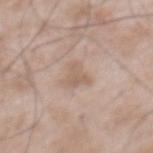<tbp_lesion>
<biopsy_status>not biopsied; imaged during a skin examination</biopsy_status>
<patient>
  <sex>male</sex>
  <age_approx>50</age_approx>
</patient>
<lighting>white-light</lighting>
<site>mid back</site>
<automated_metrics>
  <area_mm2_approx>4.0</area_mm2_approx>
  <eccentricity>0.7</eccentricity>
  <shape_asymmetry>0.5</shape_asymmetry>
  <cielab_L>59</cielab_L>
  <cielab_a>16</cielab_a>
  <cielab_b>27</cielab_b>
  <vs_skin_contrast_norm>5.5</vs_skin_contrast_norm>
  <border_irregularity_0_10>5.0</border_irregularity_0_10>
  <color_variation_0_10>1.0</color_variation_0_10>
  <peripheral_color_asymmetry>0.5</peripheral_color_asymmetry>
</automated_metrics>
<lesion_size>
  <long_diameter_mm_approx>2.5</long_diameter_mm_approx>
</lesion_size>
<image>
  <source>total-body photography crop</source>
  <field_of_view_mm>15</field_of_view_mm>
</image>
</tbp_lesion>